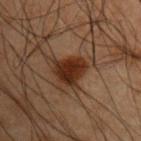Clinical impression: Imaged during a routine full-body skin examination; the lesion was not biopsied and no histopathology is available. Acquisition and patient details: Imaged with cross-polarized lighting. From the right upper arm. A male subject, aged 48–52. A lesion tile, about 15 mm wide, cut from a 3D total-body photograph. About 4 mm across. Automated tile analysis of the lesion measured an outline eccentricity of about 0.55 (0 = round, 1 = elongated) and a symmetry-axis asymmetry near 0.3. It also reported a lesion color around L≈21 a*≈17 b*≈23 in CIELAB and about 10 CIELAB-L* units darker than the surrounding skin. The software also gave a border-irregularity index near 3/10, a within-lesion color-variation index near 3/10, and peripheral color asymmetry of about 1.